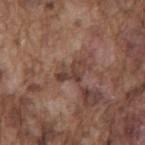The lesion was tiled from a total-body skin photograph and was not biopsied. The patient is a male aged approximately 75. A roughly 15 mm field-of-view crop from a total-body skin photograph. Located on the back. The recorded lesion diameter is about 3.5 mm. Captured under white-light illumination.